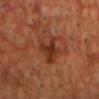biopsy_status: not biopsied; imaged during a skin examination
lesion_size:
  long_diameter_mm_approx: 4.0
patient:
  sex: male
  age_approx: 55
site: head or neck
image:
  source: total-body photography crop
  field_of_view_mm: 15